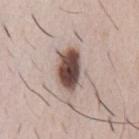follow-up=total-body-photography surveillance lesion; no biopsy | subject=male, aged 48 to 52 | body site=the front of the torso | image=total-body-photography crop, ~15 mm field of view.